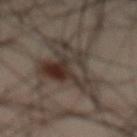notes = catalogued during a skin exam; not biopsied
image source = 15 mm crop, total-body photography
size = ~9 mm (longest diameter)
location = the abdomen
patient = male, about 50 years old